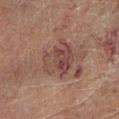Part of a total-body skin-imaging series; this lesion was reviewed on a skin check and was not flagged for biopsy.
Measured at roughly 5 mm in maximum diameter.
The subject is a male about 60 years old.
The lesion is on the left lower leg.
Cropped from a whole-body photographic skin survey; the tile spans about 15 mm.